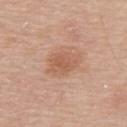This lesion was catalogued during total-body skin photography and was not selected for biopsy. About 4.5 mm across. An algorithmic analysis of the crop reported an area of roughly 9 mm², an eccentricity of roughly 0.75, and a shape-asymmetry score of about 0.2 (0 = symmetric). The software also gave a lesion-to-skin contrast of about 6 (normalized; higher = more distinct). A 15 mm crop from a total-body photograph taken for skin-cancer surveillance. A male subject, roughly 70 years of age. The lesion is located on the upper back. The tile uses white-light illumination.